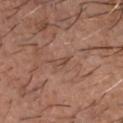{"biopsy_status": "not biopsied; imaged during a skin examination", "patient": {"sex": "male", "age_approx": 55}, "site": "head or neck", "image": {"source": "total-body photography crop", "field_of_view_mm": 15}, "lighting": "white-light", "lesion_size": {"long_diameter_mm_approx": 2.5}, "automated_metrics": {"area_mm2_approx": 2.0, "eccentricity": 0.95, "shape_asymmetry": 0.35, "border_irregularity_0_10": 4.0, "color_variation_0_10": 0.0, "peripheral_color_asymmetry": 0.0}}